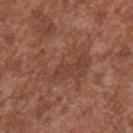The lesion was photographed on a routine skin check and not biopsied; there is no pathology result.
A male patient, aged 43–47.
On the upper back.
A roughly 15 mm field-of-view crop from a total-body skin photograph.